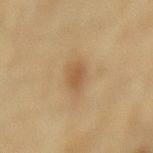Q: Was this lesion biopsied?
A: imaged on a skin check; not biopsied
Q: What lighting was used for the tile?
A: cross-polarized
Q: How was this image acquired?
A: ~15 mm crop, total-body skin-cancer survey
Q: Lesion size?
A: ≈3 mm
Q: Automated lesion metrics?
A: radial color variation of about 0.5
Q: Who is the patient?
A: female, aged 53–57
Q: Lesion location?
A: the mid back